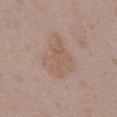biopsy status — imaged on a skin check; not biopsied
image source — 15 mm crop, total-body photography
location — the chest
subject — female, about 35 years old